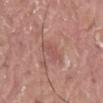| key | value |
|---|---|
| notes | no biopsy performed (imaged during a skin exam) |
| site | the left thigh |
| image-analysis metrics | a lesion area of about 5 mm², a shape eccentricity near 0.85, and two-axis asymmetry of about 0.25; a mean CIELAB color near L≈53 a*≈24 b*≈24, roughly 7 lightness units darker than nearby skin, and a normalized lesion–skin contrast near 5 |
| image source | total-body-photography crop, ~15 mm field of view |
| patient | male, aged approximately 40 |
| lesion diameter | about 3.5 mm |
| tile lighting | white-light illumination |